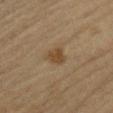notes: no biopsy performed (imaged during a skin exam)
lighting: cross-polarized
image source: ~15 mm tile from a whole-body skin photo
automated metrics: a shape eccentricity near 0.7 and two-axis asymmetry of about 0.3; a border-irregularity rating of about 2.5/10, a within-lesion color-variation index near 1.5/10, and peripheral color asymmetry of about 0.5
patient: male, roughly 65 years of age
body site: the left upper arm
size: about 2.5 mm